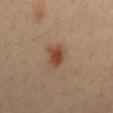Part of a total-body skin-imaging series; this lesion was reviewed on a skin check and was not flagged for biopsy.